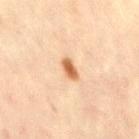<tbp_lesion>
  <biopsy_status>not biopsied; imaged during a skin examination</biopsy_status>
  <image>
    <source>total-body photography crop</source>
    <field_of_view_mm>15</field_of_view_mm>
  </image>
  <site>right thigh</site>
  <lesion_size>
    <long_diameter_mm_approx>2.5</long_diameter_mm_approx>
  </lesion_size>
  <patient>
    <sex>female</sex>
    <age_approx>45</age_approx>
  </patient>
</tbp_lesion>